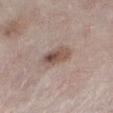Clinical summary: This is a white-light tile. Located on the left lower leg. A male subject in their 80s. Automated tile analysis of the lesion measured a lesion area of about 7 mm², a shape eccentricity near 0.85, and a shape-asymmetry score of about 0.2 (0 = symmetric). The analysis additionally found an average lesion color of about L≈51 a*≈17 b*≈23 (CIELAB), roughly 12 lightness units darker than nearby skin, and a lesion-to-skin contrast of about 8.5 (normalized; higher = more distinct). A roughly 15 mm field-of-view crop from a total-body skin photograph.